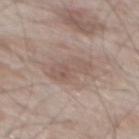The lesion was tiled from a total-body skin photograph and was not biopsied.
A male subject aged approximately 55.
Captured under white-light illumination.
From the mid back.
Automated image analysis of the tile measured a lesion area of about 9 mm² and an outline eccentricity of about 0.85 (0 = round, 1 = elongated). It also reported a border-irregularity rating of about 3.5/10, internal color variation of about 3.5 on a 0–10 scale, and radial color variation of about 1. The software also gave an automated nevus-likeness rating near 0 out of 100 and lesion-presence confidence of about 100/100.
A 15 mm crop from a total-body photograph taken for skin-cancer surveillance.
The recorded lesion diameter is about 4.5 mm.